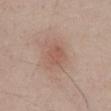The lesion was tiled from a total-body skin photograph and was not biopsied. A 15 mm crop from a total-body photograph taken for skin-cancer surveillance. The lesion is on the front of the torso. The patient is a male aged 48–52. Captured under white-light illumination.